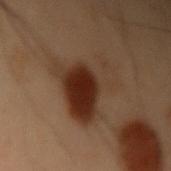image — 15 mm crop, total-body photography
lesion diameter — ~7 mm (longest diameter)
location — the left upper arm
illumination — cross-polarized
subject — male, approximately 55 years of age
TBP lesion metrics — border irregularity of about 6 on a 0–10 scale and radial color variation of about 2.5; an automated nevus-likeness rating near 95 out of 100